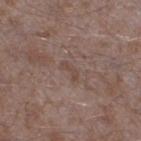Clinical impression: Imaged during a routine full-body skin examination; the lesion was not biopsied and no histopathology is available. Acquisition and patient details: On the right thigh. A region of skin cropped from a whole-body photographic capture, roughly 15 mm wide. The recorded lesion diameter is about 3 mm. A male subject, aged approximately 45. Captured under white-light illumination. The lesion-visualizer software estimated an area of roughly 3 mm², an eccentricity of roughly 0.9, and two-axis asymmetry of about 0.45. And it measured border irregularity of about 5 on a 0–10 scale, a within-lesion color-variation index near 0/10, and a peripheral color-asymmetry measure near 0. And it measured an automated nevus-likeness rating near 0 out of 100 and a lesion-detection confidence of about 85/100.